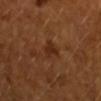This lesion was catalogued during total-body skin photography and was not selected for biopsy. Located on the right forearm. A female subject aged 53 to 57. A 15 mm close-up extracted from a 3D total-body photography capture.